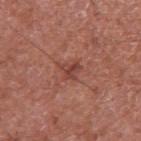Impression: The lesion was photographed on a routine skin check and not biopsied; there is no pathology result. Clinical summary: A male patient, about 65 years old. The lesion-visualizer software estimated an average lesion color of about L≈43 a*≈26 b*≈26 (CIELAB) and about 8 CIELAB-L* units darker than the surrounding skin. The analysis additionally found border irregularity of about 5 on a 0–10 scale, a color-variation rating of about 2/10, and peripheral color asymmetry of about 0.5. From the arm. This image is a 15 mm lesion crop taken from a total-body photograph.